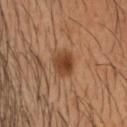Impression:
The lesion was tiled from a total-body skin photograph and was not biopsied.
Acquisition and patient details:
Automated tile analysis of the lesion measured a lesion area of about 7.5 mm² and two-axis asymmetry of about 0.2. A 15 mm crop from a total-body photograph taken for skin-cancer surveillance. About 3 mm across. The patient is a male about 45 years old. On the head or neck.